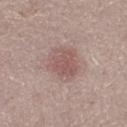workup: imaged on a skin check; not biopsied | subject: female, aged 18–22 | illumination: white-light illumination | diameter: ≈3.5 mm | acquisition: 15 mm crop, total-body photography | automated metrics: a footprint of about 8.5 mm² and two-axis asymmetry of about 0.25 | location: the left lower leg.